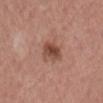biopsy_status: not biopsied; imaged during a skin examination